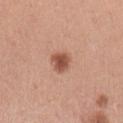| field | value |
|---|---|
| biopsy status | no biopsy performed (imaged during a skin exam) |
| size | ≈2.5 mm |
| TBP lesion metrics | an average lesion color of about L≈53 a*≈24 b*≈30 (CIELAB), about 13 CIELAB-L* units darker than the surrounding skin, and a normalized border contrast of about 9; a border-irregularity index near 2/10 and a within-lesion color-variation index near 3/10; an automated nevus-likeness rating near 100 out of 100 |
| patient | female, aged approximately 30 |
| illumination | white-light illumination |
| acquisition | 15 mm crop, total-body photography |
| anatomic site | the left upper arm |